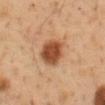Case summary:
- notes — catalogued during a skin exam; not biopsied
- image — ~15 mm crop, total-body skin-cancer survey
- size — about 4 mm
- patient — male, aged 48–52
- location — the abdomen
- illumination — cross-polarized